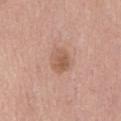| key | value |
|---|---|
| follow-up | total-body-photography surveillance lesion; no biopsy |
| patient | male, roughly 75 years of age |
| TBP lesion metrics | an area of roughly 6.5 mm² and two-axis asymmetry of about 0.15; a mean CIELAB color near L≈57 a*≈21 b*≈29, about 9 CIELAB-L* units darker than the surrounding skin, and a normalized lesion–skin contrast near 6.5 |
| location | the front of the torso |
| imaging modality | total-body-photography crop, ~15 mm field of view |
| lesion size | ≈3.5 mm |